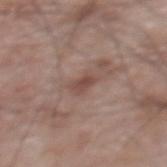{
  "biopsy_status": "not biopsied; imaged during a skin examination",
  "lesion_size": {
    "long_diameter_mm_approx": 3.0
  },
  "lighting": "white-light",
  "site": "upper back",
  "patient": {
    "sex": "male",
    "age_approx": 70
  },
  "image": {
    "source": "total-body photography crop",
    "field_of_view_mm": 15
  }
}